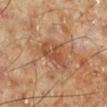Case summary:
* biopsy status: catalogued during a skin exam; not biopsied
* image: ~15 mm crop, total-body skin-cancer survey
* tile lighting: cross-polarized illumination
* site: the left lower leg
* size: ~4 mm (longest diameter)
* subject: male, aged 68–72